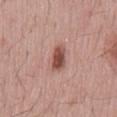{"biopsy_status": "not biopsied; imaged during a skin examination", "image": {"source": "total-body photography crop", "field_of_view_mm": 15}, "patient": {"sex": "male", "age_approx": 65}, "lesion_size": {"long_diameter_mm_approx": 3.0}, "lighting": "white-light", "site": "mid back"}